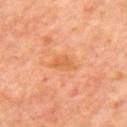Q: Was this lesion biopsied?
A: catalogued during a skin exam; not biopsied
Q: What is the imaging modality?
A: 15 mm crop, total-body photography
Q: What is the anatomic site?
A: the upper back
Q: Patient demographics?
A: male, aged 58–62
Q: How was the tile lit?
A: cross-polarized